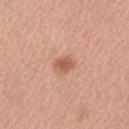Captured during whole-body skin photography for melanoma surveillance; the lesion was not biopsied. A male patient, aged 53–57. A roughly 15 mm field-of-view crop from a total-body skin photograph. On the left upper arm. Automated image analysis of the tile measured border irregularity of about 1.5 on a 0–10 scale and a color-variation rating of about 2.5/10. Longest diameter approximately 2.5 mm.